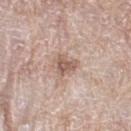<tbp_lesion>
<biopsy_status>not biopsied; imaged during a skin examination</biopsy_status>
<site>leg</site>
<image>
  <source>total-body photography crop</source>
  <field_of_view_mm>15</field_of_view_mm>
</image>
<patient>
  <sex>female</sex>
  <age_approx>60</age_approx>
</patient>
</tbp_lesion>